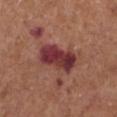Impression:
Part of a total-body skin-imaging series; this lesion was reviewed on a skin check and was not flagged for biopsy.
Image and clinical context:
The lesion's longest dimension is about 5.5 mm. Located on the left lower leg. A 15 mm close-up tile from a total-body photography series done for melanoma screening. Imaged with white-light lighting. A male patient, roughly 75 years of age. An algorithmic analysis of the crop reported an average lesion color of about L≈37 a*≈28 b*≈21 (CIELAB), a lesion–skin lightness drop of about 13, and a normalized lesion–skin contrast near 12.